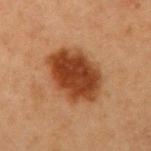Impression:
Part of a total-body skin-imaging series; this lesion was reviewed on a skin check and was not flagged for biopsy.
Image and clinical context:
The subject is a female aged approximately 40. An algorithmic analysis of the crop reported border irregularity of about 1.5 on a 0–10 scale and a peripheral color-asymmetry measure near 1.5. It also reported a detector confidence of about 100 out of 100 that the crop contains a lesion. The tile uses cross-polarized illumination. The lesion's longest dimension is about 6.5 mm. The lesion is on the arm. Cropped from a total-body skin-imaging series; the visible field is about 15 mm.